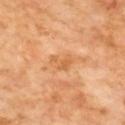| field | value |
|---|---|
| biopsy status | imaged on a skin check; not biopsied |
| image | ~15 mm crop, total-body skin-cancer survey |
| anatomic site | the upper back |
| lighting | cross-polarized |